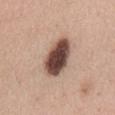workup: no biopsy performed (imaged during a skin exam)
image: 15 mm crop, total-body photography
patient: male, aged 58 to 62
anatomic site: the abdomen
diameter: ≈5.5 mm
illumination: white-light illumination
automated lesion analysis: a lesion area of about 13 mm², a shape eccentricity near 0.8, and a shape-asymmetry score of about 0.15 (0 = symmetric); a lesion color around L≈46 a*≈19 b*≈24 in CIELAB and about 23 CIELAB-L* units darker than the surrounding skin